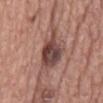The lesion was tiled from a total-body skin photograph and was not biopsied.